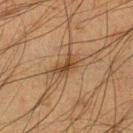Impression: Recorded during total-body skin imaging; not selected for excision or biopsy. Image and clinical context: On the left lower leg. This is a cross-polarized tile. A lesion tile, about 15 mm wide, cut from a 3D total-body photograph. The patient is a male about 35 years old. About 3 mm across.